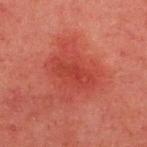{"biopsy_status": "not biopsied; imaged during a skin examination", "lesion_size": {"long_diameter_mm_approx": 5.0}, "site": "upper back", "lighting": "cross-polarized", "automated_metrics": {"area_mm2_approx": 9.5, "eccentricity": 0.9, "shape_asymmetry": 0.25, "border_irregularity_0_10": 3.0, "peripheral_color_asymmetry": 0.5, "nevus_likeness_0_100": 0, "lesion_detection_confidence_0_100": 100}, "patient": {"sex": "male", "age_approx": 45}, "image": {"source": "total-body photography crop", "field_of_view_mm": 15}}